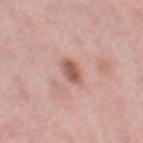Clinical impression:
Part of a total-body skin-imaging series; this lesion was reviewed on a skin check and was not flagged for biopsy.
Image and clinical context:
Imaged with white-light lighting. A female patient, aged around 50. This image is a 15 mm lesion crop taken from a total-body photograph. The lesion is located on the right thigh.